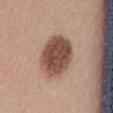{"biopsy_status": "not biopsied; imaged during a skin examination", "patient": {"sex": "female", "age_approx": 25}, "automated_metrics": {"area_mm2_approx": 19.0, "eccentricity": 0.7, "shape_asymmetry": 0.1, "border_irregularity_0_10": 1.0, "peripheral_color_asymmetry": 1.5, "lesion_detection_confidence_0_100": 100}, "lighting": "white-light", "image": {"source": "total-body photography crop", "field_of_view_mm": 15}, "site": "mid back", "lesion_size": {"long_diameter_mm_approx": 6.0}}